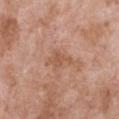Part of a total-body skin-imaging series; this lesion was reviewed on a skin check and was not flagged for biopsy. A female patient in their mid-70s. Located on the chest. A 15 mm close-up tile from a total-body photography series done for melanoma screening. Imaged with white-light lighting.